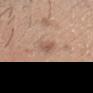Clinical impression:
The lesion was tiled from a total-body skin photograph and was not biopsied.
Acquisition and patient details:
Automated tile analysis of the lesion measured an area of roughly 3.5 mm², an outline eccentricity of about 0.8 (0 = round, 1 = elongated), and a symmetry-axis asymmetry near 0.35. From the head or neck. Measured at roughly 2.5 mm in maximum diameter. The tile uses white-light illumination. A 15 mm crop from a total-body photograph taken for skin-cancer surveillance. A male patient aged 28 to 32.